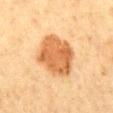Clinical impression:
Captured during whole-body skin photography for melanoma surveillance; the lesion was not biopsied.
Clinical summary:
A 15 mm close-up tile from a total-body photography series done for melanoma screening. On the mid back. A female subject, approximately 50 years of age. This is a cross-polarized tile. The lesion-visualizer software estimated a lesion color around L≈55 a*≈22 b*≈38 in CIELAB, a lesion–skin lightness drop of about 13, and a normalized lesion–skin contrast near 9.